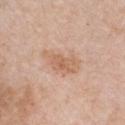| key | value |
|---|---|
| workup | imaged on a skin check; not biopsied |
| size | ~4.5 mm (longest diameter) |
| automated metrics | a nevus-likeness score of about 0/100 and a lesion-detection confidence of about 100/100 |
| acquisition | ~15 mm crop, total-body skin-cancer survey |
| site | the chest |
| subject | female, aged around 45 |